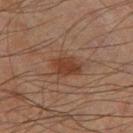A region of skin cropped from a whole-body photographic capture, roughly 15 mm wide.
The lesion-visualizer software estimated a normalized lesion–skin contrast near 8. The analysis additionally found a border-irregularity index near 2/10, a within-lesion color-variation index near 2/10, and radial color variation of about 0.5. It also reported an automated nevus-likeness rating near 80 out of 100.
The lesion is on the left thigh.
The tile uses cross-polarized illumination.
A male subject aged approximately 70.
About 3.5 mm across.